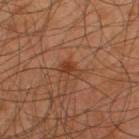Recorded during total-body skin imaging; not selected for excision or biopsy. Automated image analysis of the tile measured a border-irregularity rating of about 3/10, a within-lesion color-variation index near 2/10, and a peripheral color-asymmetry measure near 0.5. A 15 mm crop from a total-body photograph taken for skin-cancer surveillance. The patient is a male approximately 45 years of age. The tile uses cross-polarized illumination. Approximately 2.5 mm at its widest. On the upper back.